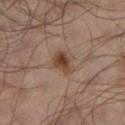Part of a total-body skin-imaging series; this lesion was reviewed on a skin check and was not flagged for biopsy. A male subject roughly 65 years of age. An algorithmic analysis of the crop reported a mean CIELAB color near L≈39 a*≈17 b*≈26, about 11 CIELAB-L* units darker than the surrounding skin, and a normalized lesion–skin contrast near 9.5. The software also gave border irregularity of about 2 on a 0–10 scale, a color-variation rating of about 4.5/10, and peripheral color asymmetry of about 1.5. The lesion's longest dimension is about 3 mm. The lesion is on the left lower leg. Imaged with cross-polarized lighting. This image is a 15 mm lesion crop taken from a total-body photograph.